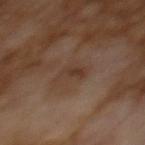<record>
  <biopsy_status>not biopsied; imaged during a skin examination</biopsy_status>
  <image>
    <source>total-body photography crop</source>
    <field_of_view_mm>15</field_of_view_mm>
  </image>
  <lighting>cross-polarized</lighting>
  <patient>
    <sex>male</sex>
    <age_approx>65</age_approx>
  </patient>
</record>